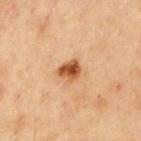Image and clinical context: Imaged with cross-polarized lighting. On the chest. Automated image analysis of the tile measured an eccentricity of roughly 0.6 and a symmetry-axis asymmetry near 0.3. And it measured a border-irregularity rating of about 2.5/10 and a color-variation rating of about 4.5/10. The analysis additionally found a classifier nevus-likeness of about 100/100 and a detector confidence of about 100 out of 100 that the crop contains a lesion. The lesion's longest dimension is about 3 mm. The subject is a male in their mid- to late 50s. This image is a 15 mm lesion crop taken from a total-body photograph.